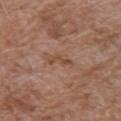<case>
  <biopsy_status>not biopsied; imaged during a skin examination</biopsy_status>
  <automated_metrics>
    <area_mm2_approx>4.5</area_mm2_approx>
    <eccentricity>0.95</eccentricity>
    <border_irregularity_0_10>5.0</border_irregularity_0_10>
    <peripheral_color_asymmetry>1.0</peripheral_color_asymmetry>
    <nevus_likeness_0_100>0</nevus_likeness_0_100>
    <lesion_detection_confidence_0_100>95</lesion_detection_confidence_0_100>
  </automated_metrics>
  <lighting>white-light</lighting>
  <lesion_size>
    <long_diameter_mm_approx>4.0</long_diameter_mm_approx>
  </lesion_size>
  <patient>
    <sex>male</sex>
    <age_approx>70</age_approx>
  </patient>
  <site>left upper arm</site>
  <image>
    <source>total-body photography crop</source>
    <field_of_view_mm>15</field_of_view_mm>
  </image>
</case>